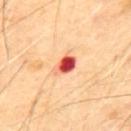Clinical impression: This lesion was catalogued during total-body skin photography and was not selected for biopsy. Image and clinical context: On the mid back. The patient is a male aged approximately 70. Cropped from a whole-body photographic skin survey; the tile spans about 15 mm.